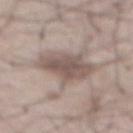Impression: Part of a total-body skin-imaging series; this lesion was reviewed on a skin check and was not flagged for biopsy.